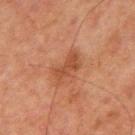Q: Was this lesion biopsied?
A: no biopsy performed (imaged during a skin exam)
Q: Lesion size?
A: ~4.5 mm (longest diameter)
Q: Patient demographics?
A: male, in their mid-60s
Q: What is the imaging modality?
A: 15 mm crop, total-body photography
Q: Where on the body is the lesion?
A: the chest
Q: How was the tile lit?
A: cross-polarized
Q: What did automated image analysis measure?
A: a mean CIELAB color near L≈39 a*≈22 b*≈29, roughly 7 lightness units darker than nearby skin, and a normalized lesion–skin contrast near 6.5; a within-lesion color-variation index near 3/10 and a peripheral color-asymmetry measure near 1